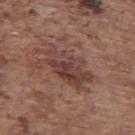The lesion is located on the upper back. The patient is a female aged around 65. The lesion's longest dimension is about 7 mm. A 15 mm close-up tile from a total-body photography series done for melanoma screening. This is a white-light tile.